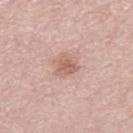<tbp_lesion>
<biopsy_status>not biopsied; imaged during a skin examination</biopsy_status>
<image>
  <source>total-body photography crop</source>
  <field_of_view_mm>15</field_of_view_mm>
</image>
<lighting>white-light</lighting>
<patient>
  <sex>female</sex>
  <age_approx>65</age_approx>
</patient>
<site>right thigh</site>
<lesion_size>
  <long_diameter_mm_approx>2.5</long_diameter_mm_approx>
</lesion_size>
</tbp_lesion>